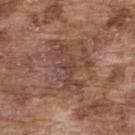Imaged during a routine full-body skin examination; the lesion was not biopsied and no histopathology is available. Automated tile analysis of the lesion measured a lesion color around L≈43 a*≈20 b*≈25 in CIELAB and a lesion–skin lightness drop of about 7. The analysis additionally found a border-irregularity index near 8/10, a color-variation rating of about 2/10, and peripheral color asymmetry of about 0.5. The software also gave a classifier nevus-likeness of about 0/100 and a lesion-detection confidence of about 80/100. This image is a 15 mm lesion crop taken from a total-body photograph. The subject is a male in their mid-70s. On the back. Captured under white-light illumination. The lesion's longest dimension is about 5.5 mm.